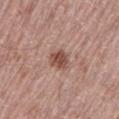Findings:
– illumination: white-light
– lesion diameter: about 3 mm
– location: the left thigh
– image source: 15 mm crop, total-body photography
– TBP lesion metrics: a lesion-to-skin contrast of about 8.5 (normalized; higher = more distinct); an automated nevus-likeness rating near 90 out of 100 and a lesion-detection confidence of about 100/100
– subject: male, aged 68 to 72